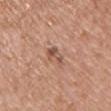| field | value |
|---|---|
| workup | total-body-photography surveillance lesion; no biopsy |
| subject | female, in their 40s |
| anatomic site | the chest |
| image | 15 mm crop, total-body photography |